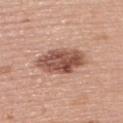Q: Lesion size?
A: ~6 mm (longest diameter)
Q: What is the imaging modality?
A: 15 mm crop, total-body photography
Q: What are the patient's age and sex?
A: female, aged around 60
Q: Lesion location?
A: the back
Q: What did automated image analysis measure?
A: an area of roughly 15 mm² and two-axis asymmetry of about 0.15; border irregularity of about 2.5 on a 0–10 scale and a within-lesion color-variation index near 5.5/10